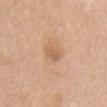Q: Is there a histopathology result?
A: no biopsy performed (imaged during a skin exam)
Q: What did automated image analysis measure?
A: border irregularity of about 2 on a 0–10 scale, a within-lesion color-variation index near 1.5/10, and radial color variation of about 0.5
Q: Patient demographics?
A: female, approximately 70 years of age
Q: How was the tile lit?
A: white-light illumination
Q: How large is the lesion?
A: ≈2.5 mm
Q: How was this image acquired?
A: ~15 mm crop, total-body skin-cancer survey
Q: Where on the body is the lesion?
A: the chest